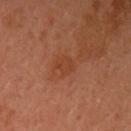biopsy status = no biopsy performed (imaged during a skin exam) | subject = female, aged around 35 | body site = the left upper arm | lesion diameter = ≈2.5 mm | imaging modality = 15 mm crop, total-body photography | illumination = cross-polarized.